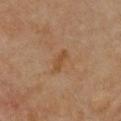Captured during whole-body skin photography for melanoma surveillance; the lesion was not biopsied.
Cropped from a total-body skin-imaging series; the visible field is about 15 mm.
Imaged with cross-polarized lighting.
The patient is a female about 70 years old.
Automated image analysis of the tile measured a lesion color around L≈42 a*≈17 b*≈31 in CIELAB and about 6 CIELAB-L* units darker than the surrounding skin. And it measured a within-lesion color-variation index near 1.5/10 and peripheral color asymmetry of about 0.5.
Located on the front of the torso.